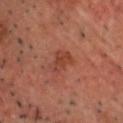Q: Is there a histopathology result?
A: total-body-photography surveillance lesion; no biopsy
Q: Where on the body is the lesion?
A: the head or neck
Q: Lesion size?
A: about 3 mm
Q: How was this image acquired?
A: ~15 mm tile from a whole-body skin photo
Q: Who is the patient?
A: male, about 50 years old
Q: How was the tile lit?
A: cross-polarized
Q: Automated lesion metrics?
A: a mean CIELAB color near L≈31 a*≈21 b*≈23 and a normalized border contrast of about 6; a border-irregularity index near 3/10 and a within-lesion color-variation index near 3/10; a nevus-likeness score of about 15/100